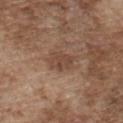| key | value |
|---|---|
| notes | no biopsy performed (imaged during a skin exam) |
| body site | the chest |
| TBP lesion metrics | roughly 8 lightness units darker than nearby skin and a normalized border contrast of about 6.5 |
| image source | 15 mm crop, total-body photography |
| patient | male, approximately 75 years of age |